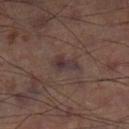Case summary:
* biopsy status · imaged on a skin check; not biopsied
* anatomic site · the left lower leg
* diameter · about 3 mm
* image source · ~15 mm tile from a whole-body skin photo
* tile lighting · cross-polarized